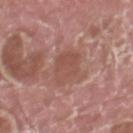Q: How was this image acquired?
A: ~15 mm tile from a whole-body skin photo
Q: What lighting was used for the tile?
A: white-light
Q: What are the patient's age and sex?
A: male, about 40 years old
Q: What is the lesion's diameter?
A: about 4.5 mm
Q: Where on the body is the lesion?
A: the left thigh